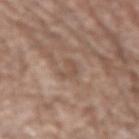workup = no biopsy performed (imaged during a skin exam) | patient = male, about 70 years old | lighting = white-light illumination | image source = 15 mm crop, total-body photography | location = the left forearm.